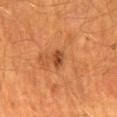<case>
  <biopsy_status>not biopsied; imaged during a skin examination</biopsy_status>
  <site>mid back</site>
  <patient>
    <sex>male</sex>
    <age_approx>65</age_approx>
  </patient>
  <image>
    <source>total-body photography crop</source>
    <field_of_view_mm>15</field_of_view_mm>
  </image>
</case>